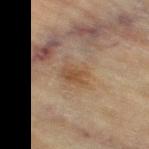workup = total-body-photography surveillance lesion; no biopsy | patient = female, approximately 80 years of age | anatomic site = the right thigh | diameter = ≈3 mm | image source = ~15 mm crop, total-body skin-cancer survey.